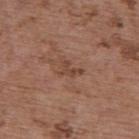The lesion was photographed on a routine skin check and not biopsied; there is no pathology result. Automated tile analysis of the lesion measured an area of roughly 3.5 mm² and two-axis asymmetry of about 0.6. And it measured a lesion color around L≈44 a*≈20 b*≈28 in CIELAB, a lesion–skin lightness drop of about 7, and a normalized border contrast of about 6. The software also gave an automated nevus-likeness rating near 0 out of 100. Captured under white-light illumination. A female patient roughly 65 years of age. Located on the upper back. Longest diameter approximately 2.5 mm. Cropped from a total-body skin-imaging series; the visible field is about 15 mm.